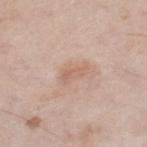Q: Patient demographics?
A: male, approximately 60 years of age
Q: What is the lesion's diameter?
A: ~3 mm (longest diameter)
Q: Illumination type?
A: white-light
Q: What kind of image is this?
A: ~15 mm crop, total-body skin-cancer survey
Q: Where on the body is the lesion?
A: the right lower leg
Q: Automated lesion metrics?
A: lesion-presence confidence of about 100/100